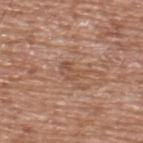workup — total-body-photography surveillance lesion; no biopsy | image — ~15 mm tile from a whole-body skin photo | automated metrics — a lesion–skin lightness drop of about 7 and a normalized lesion–skin contrast near 5.5; an automated nevus-likeness rating near 0 out of 100 and lesion-presence confidence of about 100/100 | patient — male, in their mid- to late 70s | body site — the upper back | lighting — white-light illumination | size — about 3 mm.